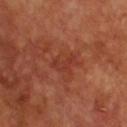Clinical impression:
The lesion was photographed on a routine skin check and not biopsied; there is no pathology result.
Acquisition and patient details:
The lesion is on the chest. A lesion tile, about 15 mm wide, cut from a 3D total-body photograph. The recorded lesion diameter is about 3.5 mm. The subject is a male about 65 years old. This is a cross-polarized tile.